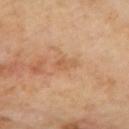Case summary:
– workup — no biopsy performed (imaged during a skin exam)
– image source — ~15 mm tile from a whole-body skin photo
– location — the mid back
– image-analysis metrics — an area of roughly 3.5 mm², an outline eccentricity of about 0.85 (0 = round, 1 = elongated), and two-axis asymmetry of about 0.3; a border-irregularity rating of about 3/10, a color-variation rating of about 1.5/10, and a peripheral color-asymmetry measure near 0.5
– diameter — about 3 mm